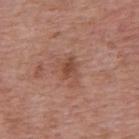Case summary:
– follow-up · catalogued during a skin exam; not biopsied
– lighting · white-light illumination
– automated lesion analysis · a lesion area of about 6.5 mm² and an eccentricity of roughly 0.8; a border-irregularity rating of about 4.5/10 and a color-variation rating of about 3.5/10; a classifier nevus-likeness of about 0/100 and a detector confidence of about 100 out of 100 that the crop contains a lesion
– subject · female, aged 38 to 42
– body site · the back
– image · ~15 mm tile from a whole-body skin photo
– lesion size · ≈3.5 mm